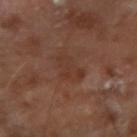Findings:
- biopsy status · no biopsy performed (imaged during a skin exam)
- illumination · cross-polarized
- lesion size · ≈4 mm
- imaging modality · total-body-photography crop, ~15 mm field of view
- automated metrics · border irregularity of about 4 on a 0–10 scale and peripheral color asymmetry of about 1
- subject · male, approximately 65 years of age
- body site · the right upper arm